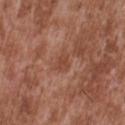Captured during whole-body skin photography for melanoma surveillance; the lesion was not biopsied.
Approximately 3 mm at its widest.
An algorithmic analysis of the crop reported border irregularity of about 3.5 on a 0–10 scale and radial color variation of about 0.5.
The subject is a male aged approximately 45.
A roughly 15 mm field-of-view crop from a total-body skin photograph.
The tile uses white-light illumination.
On the upper back.